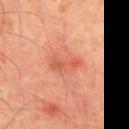Impression: Imaged during a routine full-body skin examination; the lesion was not biopsied and no histopathology is available. Context: The recorded lesion diameter is about 3.5 mm. This image is a 15 mm lesion crop taken from a total-body photograph. A male subject roughly 45 years of age. This is a cross-polarized tile. On the back.